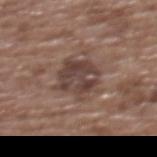Impression:
Captured during whole-body skin photography for melanoma surveillance; the lesion was not biopsied.
Background:
A female patient roughly 75 years of age. Imaged with white-light lighting. Located on the back. The recorded lesion diameter is about 5 mm. This image is a 15 mm lesion crop taken from a total-body photograph.